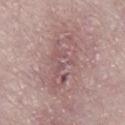{"biopsy_status": "not biopsied; imaged during a skin examination", "site": "right lower leg", "automated_metrics": {"area_mm2_approx": 16.0, "eccentricity": 0.9, "shape_asymmetry": 0.35, "cielab_L": 54, "cielab_a": 20, "cielab_b": 18, "vs_skin_darker_L": 7.0, "vs_skin_contrast_norm": 5.5, "nevus_likeness_0_100": 0, "lesion_detection_confidence_0_100": 95}, "image": {"source": "total-body photography crop", "field_of_view_mm": 15}, "lesion_size": {"long_diameter_mm_approx": 7.0}, "patient": {"sex": "female", "age_approx": 60}, "lighting": "white-light"}